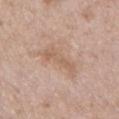Q: Was a biopsy performed?
A: imaged on a skin check; not biopsied
Q: Lesion location?
A: the chest
Q: What is the imaging modality?
A: 15 mm crop, total-body photography
Q: Lesion size?
A: about 4.5 mm
Q: Patient demographics?
A: male, about 50 years old
Q: Automated lesion metrics?
A: a border-irregularity rating of about 5/10 and radial color variation of about 0.5
Q: Illumination type?
A: white-light